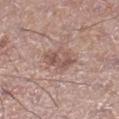Image and clinical context: Imaged with white-light lighting. From the right lower leg. The recorded lesion diameter is about 4.5 mm. A close-up tile cropped from a whole-body skin photograph, about 15 mm across. Automated tile analysis of the lesion measured a classifier nevus-likeness of about 0/100 and a lesion-detection confidence of about 100/100. A male subject, roughly 55 years of age.